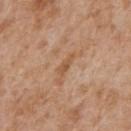– biopsy status — imaged on a skin check; not biopsied